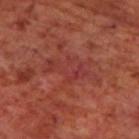Case summary:
- biopsy status: imaged on a skin check; not biopsied
- patient: male, aged 68 to 72
- size: about 6 mm
- body site: the upper back
- tile lighting: cross-polarized
- image: ~15 mm crop, total-body skin-cancer survey